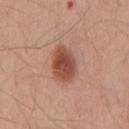- workup: imaged on a skin check; not biopsied
- location: the mid back
- automated metrics: an average lesion color of about L≈49 a*≈25 b*≈29 (CIELAB), a lesion–skin lightness drop of about 14, and a lesion-to-skin contrast of about 10 (normalized; higher = more distinct); border irregularity of about 2 on a 0–10 scale, a color-variation rating of about 4/10, and peripheral color asymmetry of about 1
- imaging modality: total-body-photography crop, ~15 mm field of view
- tile lighting: white-light illumination
- subject: male, aged 38 to 42
- diameter: ~4.5 mm (longest diameter)